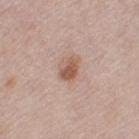Findings:
• biopsy status · no biopsy performed (imaged during a skin exam)
• subject · female, in their 60s
• image source · total-body-photography crop, ~15 mm field of view
• site · the right thigh